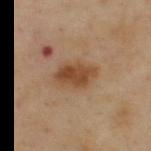Case summary:
– follow-up — catalogued during a skin exam; not biopsied
– patient — male, aged 53–57
– anatomic site — the upper back
– diameter — about 4.5 mm
– image — ~15 mm tile from a whole-body skin photo
– image-analysis metrics — a footprint of about 9.5 mm², an outline eccentricity of about 0.8 (0 = round, 1 = elongated), and a symmetry-axis asymmetry near 0.25; a nevus-likeness score of about 95/100
– lighting — cross-polarized